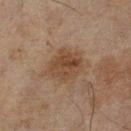<tbp_lesion>
  <biopsy_status>not biopsied; imaged during a skin examination</biopsy_status>
  <image>
    <source>total-body photography crop</source>
    <field_of_view_mm>15</field_of_view_mm>
  </image>
  <lighting>cross-polarized</lighting>
  <automated_metrics>
    <nevus_likeness_0_100>40</nevus_likeness_0_100>
    <lesion_detection_confidence_0_100>100</lesion_detection_confidence_0_100>
  </automated_metrics>
  <site>left lower leg</site>
  <patient>
    <sex>male</sex>
    <age_approx>45</age_approx>
  </patient>
</tbp_lesion>